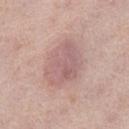  biopsy_status: not biopsied; imaged during a skin examination